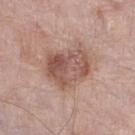Imaged during a routine full-body skin examination; the lesion was not biopsied and no histopathology is available.
A close-up tile cropped from a whole-body skin photograph, about 15 mm across.
This is a white-light tile.
The patient is a female in their mid- to late 70s.
On the right lower leg.
The total-body-photography lesion software estimated an average lesion color of about L≈54 a*≈20 b*≈25 (CIELAB) and a normalized lesion–skin contrast near 7.5.